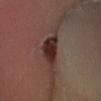This lesion was catalogued during total-body skin photography and was not selected for biopsy. An algorithmic analysis of the crop reported a footprint of about 8.5 mm², a shape eccentricity near 0.75, and two-axis asymmetry of about 0.4. And it measured a nevus-likeness score of about 85/100 and a detector confidence of about 100 out of 100 that the crop contains a lesion. The lesion's longest dimension is about 4.5 mm. From the left lower leg. A male subject, aged 38–42. The tile uses cross-polarized illumination. A 15 mm close-up tile from a total-body photography series done for melanoma screening.